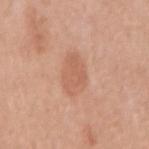{"biopsy_status": "not biopsied; imaged during a skin examination", "site": "right upper arm", "automated_metrics": {"area_mm2_approx": 9.0, "eccentricity": 0.75, "shape_asymmetry": 0.25, "border_irregularity_0_10": 2.5, "peripheral_color_asymmetry": 1.0}, "image": {"source": "total-body photography crop", "field_of_view_mm": 15}, "patient": {"sex": "female", "age_approx": 50}, "lighting": "white-light", "lesion_size": {"long_diameter_mm_approx": 4.0}}